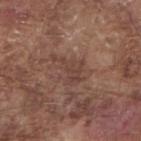Context: Automated image analysis of the tile measured a lesion area of about 6 mm², an outline eccentricity of about 0.75 (0 = round, 1 = elongated), and a symmetry-axis asymmetry near 0.7. The analysis additionally found a border-irregularity rating of about 9.5/10 and a within-lesion color-variation index near 1.5/10. And it measured a nevus-likeness score of about 0/100 and a lesion-detection confidence of about 95/100. Located on the right forearm. A close-up tile cropped from a whole-body skin photograph, about 15 mm across. The subject is a male aged approximately 75.